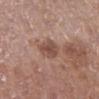biopsy status = no biopsy performed (imaged during a skin exam)
diameter = about 3 mm
subject = female, aged 73–77
site = the right lower leg
acquisition = total-body-photography crop, ~15 mm field of view
automated lesion analysis = a lesion area of about 6 mm²; a border-irregularity index near 2/10, a within-lesion color-variation index near 3.5/10, and peripheral color asymmetry of about 1; a classifier nevus-likeness of about 5/100 and a detector confidence of about 100 out of 100 that the crop contains a lesion